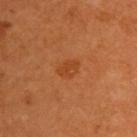  biopsy_status: not biopsied; imaged during a skin examination
  image:
    source: total-body photography crop
    field_of_view_mm: 15
  patient:
    sex: male
    age_approx: 60
  lesion_size:
    long_diameter_mm_approx: 2.5
  site: upper back
  automated_metrics:
    cielab_L: 42
    cielab_a: 28
    cielab_b: 39
    vs_skin_darker_L: 6.0
    vs_skin_contrast_norm: 5.5
    border_irregularity_0_10: 2.0
    color_variation_0_10: 2.5
    lesion_detection_confidence_0_100: 100
  lighting: cross-polarized